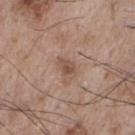Q: What is the anatomic site?
A: the chest
Q: Who is the patient?
A: male, aged 73 to 77
Q: What did automated image analysis measure?
A: a lesion color around L≈50 a*≈18 b*≈27 in CIELAB, a lesion–skin lightness drop of about 9, and a normalized lesion–skin contrast near 7; a nevus-likeness score of about 0/100 and a lesion-detection confidence of about 100/100
Q: Illumination type?
A: white-light illumination
Q: What is the imaging modality?
A: ~15 mm tile from a whole-body skin photo
Q: What is the lesion's diameter?
A: ~2.5 mm (longest diameter)